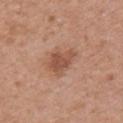follow-up — no biopsy performed (imaged during a skin exam); patient — female, aged 28 to 32; acquisition — total-body-photography crop, ~15 mm field of view; lighting — white-light illumination; body site — the back; automated lesion analysis — border irregularity of about 3 on a 0–10 scale and a within-lesion color-variation index near 2.5/10; lesion size — ≈3.5 mm.